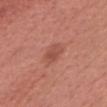Part of a total-body skin-imaging series; this lesion was reviewed on a skin check and was not flagged for biopsy. A close-up tile cropped from a whole-body skin photograph, about 15 mm across. The tile uses white-light illumination. Approximately 3 mm at its widest. A female subject, aged 63 to 67. From the head or neck. Automated tile analysis of the lesion measured an average lesion color of about L≈51 a*≈27 b*≈29 (CIELAB), about 8 CIELAB-L* units darker than the surrounding skin, and a normalized lesion–skin contrast near 5.5. It also reported a peripheral color-asymmetry measure near 1. It also reported a nevus-likeness score of about 70/100 and lesion-presence confidence of about 100/100.